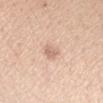image source: 15 mm crop, total-body photography
automated metrics: an area of roughly 4 mm², a shape eccentricity near 0.6, and a symmetry-axis asymmetry near 0.25; an average lesion color of about L≈68 a*≈19 b*≈28 (CIELAB) and a normalized lesion–skin contrast near 5.5; border irregularity of about 2.5 on a 0–10 scale, a color-variation rating of about 2/10, and peripheral color asymmetry of about 0.5
site: the right forearm
lesion size: ~2.5 mm (longest diameter)
subject: female, in their mid- to late 20s
tile lighting: white-light